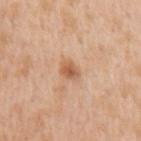<lesion>
<biopsy_status>not biopsied; imaged during a skin examination</biopsy_status>
<lighting>white-light</lighting>
<automated_metrics>
  <cielab_L>59</cielab_L>
  <cielab_a>22</cielab_a>
  <cielab_b>35</cielab_b>
  <vs_skin_darker_L>11.0</vs_skin_darker_L>
  <vs_skin_contrast_norm>7.5</vs_skin_contrast_norm>
  <border_irregularity_0_10>2.5</border_irregularity_0_10>
  <color_variation_0_10>3.5</color_variation_0_10>
  <peripheral_color_asymmetry>1.0</peripheral_color_asymmetry>
</automated_metrics>
<patient>
  <sex>female</sex>
  <age_approx>55</age_approx>
</patient>
<site>arm</site>
<image>
  <source>total-body photography crop</source>
  <field_of_view_mm>15</field_of_view_mm>
</image>
<lesion_size>
  <long_diameter_mm_approx>2.5</long_diameter_mm_approx>
</lesion_size>
</lesion>